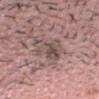Notes:
* notes — catalogued during a skin exam; not biopsied
* imaging modality — ~15 mm tile from a whole-body skin photo
* location — the head or neck
* lighting — white-light
* patient — male, aged 28–32